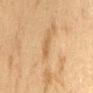| field | value |
|---|---|
| biopsy status | imaged on a skin check; not biopsied |
| site | the lower back |
| acquisition | ~15 mm tile from a whole-body skin photo |
| subject | female, aged 53 to 57 |